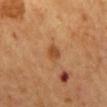Part of a total-body skin-imaging series; this lesion was reviewed on a skin check and was not flagged for biopsy. Captured under cross-polarized illumination. The lesion's longest dimension is about 2.5 mm. A male patient, in their mid- to late 60s. Located on the mid back. Cropped from a whole-body photographic skin survey; the tile spans about 15 mm.